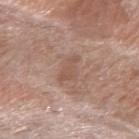Q: Was a biopsy performed?
A: total-body-photography surveillance lesion; no biopsy
Q: Where on the body is the lesion?
A: the right forearm
Q: Who is the patient?
A: female, approximately 70 years of age
Q: What kind of image is this?
A: ~15 mm crop, total-body skin-cancer survey